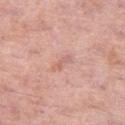Clinical impression: The lesion was tiled from a total-body skin photograph and was not biopsied. Background: The patient is a female aged 58 to 62. A 15 mm close-up extracted from a 3D total-body photography capture. The lesion is on the left thigh.